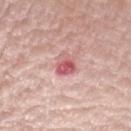| field | value |
|---|---|
| biopsy status | imaged on a skin check; not biopsied |
| location | the left upper arm |
| subject | male, aged around 85 or older |
| image-analysis metrics | an area of roughly 4.5 mm² and an eccentricity of roughly 0.6; lesion-presence confidence of about 100/100 |
| image | ~15 mm crop, total-body skin-cancer survey |